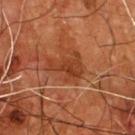Findings:
– follow-up · no biopsy performed (imaged during a skin exam)
– image source · total-body-photography crop, ~15 mm field of view
– subject · male, aged 53 to 57
– body site · the chest
– lesion size · ≈4.5 mm
– TBP lesion metrics · an area of roughly 11 mm², an outline eccentricity of about 0.65 (0 = round, 1 = elongated), and a shape-asymmetry score of about 0.5 (0 = symmetric); a border-irregularity index near 5.5/10 and a color-variation rating of about 3/10; a classifier nevus-likeness of about 0/100 and a detector confidence of about 100 out of 100 that the crop contains a lesion